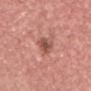Q: Is there a histopathology result?
A: no biopsy performed (imaged during a skin exam)
Q: What is the anatomic site?
A: the head or neck
Q: How was the tile lit?
A: white-light illumination
Q: How was this image acquired?
A: total-body-photography crop, ~15 mm field of view
Q: How large is the lesion?
A: ≈3 mm
Q: Patient demographics?
A: female, roughly 40 years of age